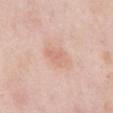* workup: imaged on a skin check; not biopsied
* anatomic site: the front of the torso
* tile lighting: white-light
* automated lesion analysis: a border-irregularity rating of about 3.5/10 and a within-lesion color-variation index near 1.5/10; an automated nevus-likeness rating near 70 out of 100 and lesion-presence confidence of about 100/100
* subject: male, approximately 55 years of age
* lesion size: about 3 mm
* image: 15 mm crop, total-body photography